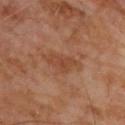workup=catalogued during a skin exam; not biopsied | anatomic site=the upper back | illumination=cross-polarized | imaging modality=~15 mm crop, total-body skin-cancer survey | subject=male, in their 70s | lesion diameter=~5 mm (longest diameter).